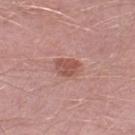Findings:
- notes — imaged on a skin check; not biopsied
- patient — male, roughly 50 years of age
- image source — ~15 mm crop, total-body skin-cancer survey
- body site — the left thigh